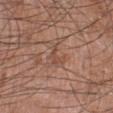Case summary:
* notes — imaged on a skin check; not biopsied
* subject — male, about 60 years old
* lesion size — ≈3 mm
* imaging modality — ~15 mm crop, total-body skin-cancer survey
* lighting — white-light illumination
* site — the leg